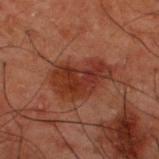subject: male, aged 48 to 52 | body site: the upper back | illumination: cross-polarized illumination | acquisition: ~15 mm tile from a whole-body skin photo | automated metrics: about 7 CIELAB-L* units darker than the surrounding skin and a normalized lesion–skin contrast near 8; a classifier nevus-likeness of about 55/100 | diameter: ~6.5 mm (longest diameter).